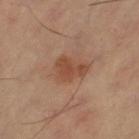biopsy_status: not biopsied; imaged during a skin examination
lighting: cross-polarized
site: left thigh
lesion_size:
  long_diameter_mm_approx: 4.0
image:
  source: total-body photography crop
  field_of_view_mm: 15
automated_metrics:
  area_mm2_approx: 9.0
  eccentricity: 0.65
  shape_asymmetry: 0.3
  nevus_likeness_0_100: 60
  lesion_detection_confidence_0_100: 100